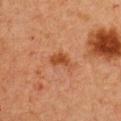lesion diameter = ~3 mm (longest diameter)
location = the chest
subject = female, approximately 40 years of age
image-analysis metrics = a lesion color around L≈40 a*≈24 b*≈34 in CIELAB and a normalized border contrast of about 8; a border-irregularity index near 4/10, a color-variation rating of about 1.5/10, and radial color variation of about 0.5
acquisition = total-body-photography crop, ~15 mm field of view